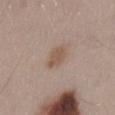Assessment:
This lesion was catalogued during total-body skin photography and was not selected for biopsy.
Image and clinical context:
The patient is a male aged approximately 55. Captured under white-light illumination. From the lower back. A roughly 15 mm field-of-view crop from a total-body skin photograph.